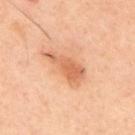The lesion was tiled from a total-body skin photograph and was not biopsied. The patient is a male aged 38 to 42. A region of skin cropped from a whole-body photographic capture, roughly 15 mm wide. Captured under cross-polarized illumination. The lesion is on the back.